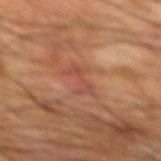workup: total-body-photography surveillance lesion; no biopsy
subject: male, aged around 65
TBP lesion metrics: a lesion area of about 4.5 mm², an eccentricity of roughly 0.85, and a shape-asymmetry score of about 0.5 (0 = symmetric); an average lesion color of about L≈38 a*≈21 b*≈25 (CIELAB), about 5 CIELAB-L* units darker than the surrounding skin, and a normalized lesion–skin contrast near 5
imaging modality: 15 mm crop, total-body photography
size: ≈3.5 mm
location: the mid back